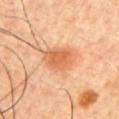biopsy status — no biopsy performed (imaged during a skin exam) | body site — the chest | subject — male, aged 43 to 47 | lighting — cross-polarized | image source — total-body-photography crop, ~15 mm field of view.